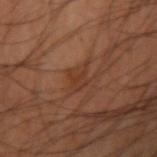Imaged during a routine full-body skin examination; the lesion was not biopsied and no histopathology is available.
Longest diameter approximately 3 mm.
Captured under cross-polarized illumination.
Cropped from a total-body skin-imaging series; the visible field is about 15 mm.
The lesion is on the right upper arm.
A male subject, aged around 40.